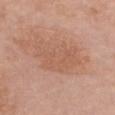{
  "biopsy_status": "not biopsied; imaged during a skin examination",
  "patient": {
    "sex": "female",
    "age_approx": 60
  },
  "lesion_size": {
    "long_diameter_mm_approx": 6.0
  },
  "image": {
    "source": "total-body photography crop",
    "field_of_view_mm": 15
  },
  "lighting": "white-light",
  "site": "chest"
}